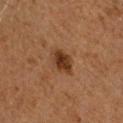follow-up: imaged on a skin check; not biopsied
imaging modality: 15 mm crop, total-body photography
lesion diameter: ≈3 mm
lighting: cross-polarized illumination
location: the head or neck
image-analysis metrics: an eccentricity of roughly 0.65 and a shape-asymmetry score of about 0.25 (0 = symmetric); a border-irregularity index near 2.5/10, a within-lesion color-variation index near 3.5/10, and peripheral color asymmetry of about 1
subject: male, aged 58–62